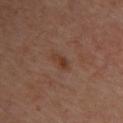biopsy status: total-body-photography surveillance lesion; no biopsy
subject: female, aged 38–42
illumination: cross-polarized
location: the back
image: total-body-photography crop, ~15 mm field of view